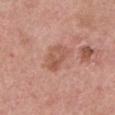biopsy status: imaged on a skin check; not biopsied
location: the front of the torso
acquisition: ~15 mm crop, total-body skin-cancer survey
subject: male, aged 58–62
illumination: white-light
image-analysis metrics: an eccentricity of roughly 0.8 and two-axis asymmetry of about 0.25; a classifier nevus-likeness of about 0/100 and a detector confidence of about 100 out of 100 that the crop contains a lesion
size: about 3.5 mm